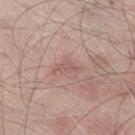{
  "biopsy_status": "not biopsied; imaged during a skin examination",
  "image": {
    "source": "total-body photography crop",
    "field_of_view_mm": 15
  },
  "patient": {
    "sex": "male",
    "age_approx": 65
  },
  "site": "leg",
  "lighting": "white-light",
  "automated_metrics": {
    "eccentricity": 0.95,
    "shape_asymmetry": 0.55,
    "cielab_L": 56,
    "cielab_a": 20,
    "cielab_b": 23,
    "vs_skin_darker_L": 7.0,
    "border_irregularity_0_10": 6.0,
    "color_variation_0_10": 0.0,
    "peripheral_color_asymmetry": 0.0,
    "nevus_likeness_0_100": 0,
    "lesion_detection_confidence_0_100": 100
  }
}